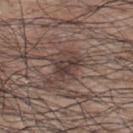Assessment: Recorded during total-body skin imaging; not selected for excision or biopsy. Context: This image is a 15 mm lesion crop taken from a total-body photograph. The lesion-visualizer software estimated a border-irregularity rating of about 4/10, a color-variation rating of about 2.5/10, and a peripheral color-asymmetry measure near 1. The software also gave a nevus-likeness score of about 15/100 and a detector confidence of about 75 out of 100 that the crop contains a lesion. Longest diameter approximately 3 mm. From the upper back. A male subject, in their 70s.